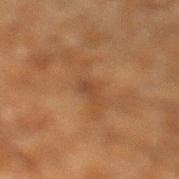  image:
    source: total-body photography crop
    field_of_view_mm: 15
  patient:
    sex: male
    age_approx: 60
  site: left lower leg
  automated_metrics:
    area_mm2_approx: 3.0
    eccentricity: 0.85
    shape_asymmetry: 0.4
    nevus_likeness_0_100: 0
    lesion_detection_confidence_0_100: 95